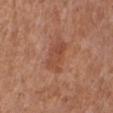The lesion is located on the left leg.
Longest diameter approximately 4 mm.
Automated tile analysis of the lesion measured lesion-presence confidence of about 100/100.
A 15 mm close-up extracted from a 3D total-body photography capture.
The tile uses white-light illumination.
A female subject, aged 63–67.